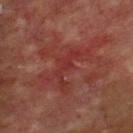Recorded during total-body skin imaging; not selected for excision or biopsy. A region of skin cropped from a whole-body photographic capture, roughly 15 mm wide. The lesion is located on the upper back. Longest diameter approximately 5 mm. The patient is a male approximately 65 years of age.